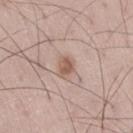| feature | finding |
|---|---|
| biopsy status | no biopsy performed (imaged during a skin exam) |
| imaging modality | 15 mm crop, total-body photography |
| diameter | ≈2.5 mm |
| site | the right thigh |
| TBP lesion metrics | a border-irregularity index near 2.5/10, internal color variation of about 2.5 on a 0–10 scale, and peripheral color asymmetry of about 1 |
| illumination | white-light illumination |
| subject | male, about 50 years old |